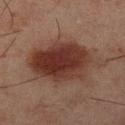Q: Was this lesion biopsied?
A: total-body-photography surveillance lesion; no biopsy
Q: Patient demographics?
A: male, in their mid- to late 40s
Q: Automated lesion metrics?
A: a nevus-likeness score of about 100/100 and lesion-presence confidence of about 100/100
Q: How was the tile lit?
A: cross-polarized illumination
Q: How large is the lesion?
A: about 9.5 mm
Q: How was this image acquired?
A: ~15 mm crop, total-body skin-cancer survey
Q: Lesion location?
A: the right lower leg